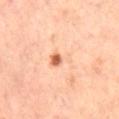- biopsy status · no biopsy performed (imaged during a skin exam)
- patient · female, aged 63 to 67
- location · the left leg
- diameter · about 3.5 mm
- imaging modality · 15 mm crop, total-body photography